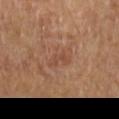Clinical impression: No biopsy was performed on this lesion — it was imaged during a full skin examination and was not determined to be concerning. Clinical summary: The lesion is on the left forearm. This image is a 15 mm lesion crop taken from a total-body photograph. Longest diameter approximately 2.5 mm. A female patient, approximately 70 years of age. The total-body-photography lesion software estimated a mean CIELAB color near L≈45 a*≈21 b*≈29 and about 6 CIELAB-L* units darker than the surrounding skin. And it measured a nevus-likeness score of about 0/100 and a detector confidence of about 100 out of 100 that the crop contains a lesion.